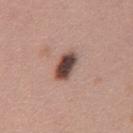Clinical impression: Captured during whole-body skin photography for melanoma surveillance; the lesion was not biopsied. Clinical summary: The lesion-visualizer software estimated a footprint of about 6.5 mm², an eccentricity of roughly 0.85, and two-axis asymmetry of about 0.2. The software also gave a mean CIELAB color near L≈45 a*≈19 b*≈22, a lesion–skin lightness drop of about 19, and a lesion-to-skin contrast of about 13.5 (normalized; higher = more distinct). A 15 mm crop from a total-body photograph taken for skin-cancer surveillance. The patient is a female aged around 40. From the chest.